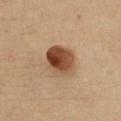  site: chest
  image:
    source: total-body photography crop
    field_of_view_mm: 15
  patient:
    sex: female
    age_approx: 35
  lesion_size:
    long_diameter_mm_approx: 4.0
  lighting: cross-polarized
  automated_metrics:
    nevus_likeness_0_100: 100
    lesion_detection_confidence_0_100: 100